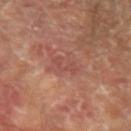{
  "biopsy_status": "not biopsied; imaged during a skin examination",
  "image": {
    "source": "total-body photography crop",
    "field_of_view_mm": 15
  },
  "lesion_size": {
    "long_diameter_mm_approx": 3.0
  },
  "site": "left forearm",
  "lighting": "cross-polarized",
  "patient": {
    "sex": "male",
    "age_approx": 65
  }
}